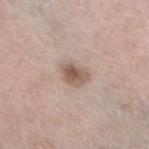This lesion was catalogued during total-body skin photography and was not selected for biopsy. This image is a 15 mm lesion crop taken from a total-body photograph. A female patient, approximately 65 years of age. Imaged with white-light lighting. From the left lower leg.